The recorded lesion diameter is about 9 mm · a lesion tile, about 15 mm wide, cut from a 3D total-body photograph · the patient is a male aged around 50 · captured under cross-polarized illumination · the lesion is located on the chest.
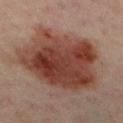Pathology:
The biopsy diagnosis was a dysplastic (Clark) nevus.